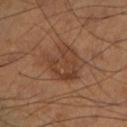{
  "biopsy_status": "not biopsied; imaged during a skin examination",
  "image": {
    "source": "total-body photography crop",
    "field_of_view_mm": 15
  },
  "site": "leg",
  "patient": {
    "sex": "male",
    "age_approx": 55
  }
}